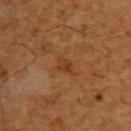Clinical impression: Recorded during total-body skin imaging; not selected for excision or biopsy. Acquisition and patient details: A region of skin cropped from a whole-body photographic capture, roughly 15 mm wide. Located on the back. The lesion's longest dimension is about 2.5 mm. This is a cross-polarized tile. The patient is a male aged approximately 60.